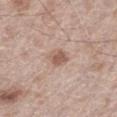No biopsy was performed on this lesion — it was imaged during a full skin examination and was not determined to be concerning.
The subject is a male aged 68 to 72.
From the left thigh.
Cropped from a whole-body photographic skin survey; the tile spans about 15 mm.